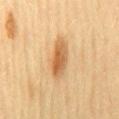{
  "biopsy_status": "not biopsied; imaged during a skin examination",
  "lesion_size": {
    "long_diameter_mm_approx": 5.0
  },
  "automated_metrics": {
    "area_mm2_approx": 9.0,
    "eccentricity": 0.9,
    "shape_asymmetry": 0.1,
    "nevus_likeness_0_100": 90,
    "lesion_detection_confidence_0_100": 100
  },
  "image": {
    "source": "total-body photography crop",
    "field_of_view_mm": 15
  },
  "patient": {
    "sex": "female",
    "age_approx": 55
  },
  "lighting": "cross-polarized",
  "site": "back"
}